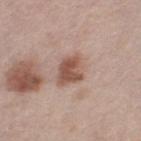biopsy status: imaged on a skin check; not biopsied | lesion diameter: ≈4 mm | illumination: white-light illumination | subject: male, roughly 65 years of age | site: the left lower leg | acquisition: 15 mm crop, total-body photography.